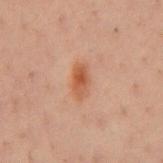A close-up tile cropped from a whole-body skin photograph, about 15 mm across.
The lesion is on the chest.
An algorithmic analysis of the crop reported an area of roughly 5 mm², an eccentricity of roughly 0.85, and two-axis asymmetry of about 0.15. And it measured roughly 8 lightness units darker than nearby skin and a normalized lesion–skin contrast near 7.5. The analysis additionally found a nevus-likeness score of about 95/100 and lesion-presence confidence of about 100/100.
A male patient, aged approximately 40.
The lesion's longest dimension is about 3.5 mm.
Captured under cross-polarized illumination.